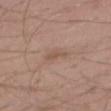Impression: Recorded during total-body skin imaging; not selected for excision or biopsy. Context: The recorded lesion diameter is about 3 mm. A region of skin cropped from a whole-body photographic capture, roughly 15 mm wide. The lesion-visualizer software estimated a lesion area of about 2.5 mm² and two-axis asymmetry of about 0.35. And it measured lesion-presence confidence of about 100/100. The tile uses white-light illumination. The lesion is on the right thigh. A male subject, aged 18 to 22.